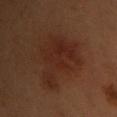The lesion was photographed on a routine skin check and not biopsied; there is no pathology result.
Imaged with cross-polarized lighting.
On the arm.
The patient is a female aged 48–52.
Cropped from a whole-body photographic skin survey; the tile spans about 15 mm.
The lesion's longest dimension is about 6 mm.